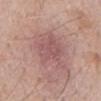The lesion was tiled from a total-body skin photograph and was not biopsied.
A lesion tile, about 15 mm wide, cut from a 3D total-body photograph.
Located on the mid back.
The patient is a male aged approximately 60.
This is a white-light tile.
The recorded lesion diameter is about 7 mm.